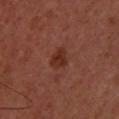This lesion was catalogued during total-body skin photography and was not selected for biopsy. Cropped from a whole-body photographic skin survey; the tile spans about 15 mm. Captured under cross-polarized illumination. A female patient aged 38 to 42. The lesion is located on the upper back.